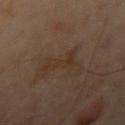<tbp_lesion>
  <biopsy_status>not biopsied; imaged during a skin examination</biopsy_status>
  <automated_metrics>
    <area_mm2_approx>5.0</area_mm2_approx>
    <shape_asymmetry>0.55</shape_asymmetry>
    <nevus_likeness_0_100>0</nevus_likeness_0_100>
    <lesion_detection_confidence_0_100>100</lesion_detection_confidence_0_100>
  </automated_metrics>
  <lighting>cross-polarized</lighting>
  <patient>
    <sex>male</sex>
    <age_approx>50</age_approx>
  </patient>
  <lesion_size>
    <long_diameter_mm_approx>4.0</long_diameter_mm_approx>
  </lesion_size>
  <site>left arm</site>
  <image>
    <source>total-body photography crop</source>
    <field_of_view_mm>15</field_of_view_mm>
  </image>
</tbp_lesion>